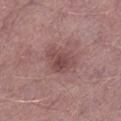Background:
Measured at roughly 4 mm in maximum diameter. A male patient, aged approximately 55. This is a white-light tile. From the left lower leg. A region of skin cropped from a whole-body photographic capture, roughly 15 mm wide.